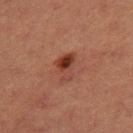  biopsy_status: not biopsied; imaged during a skin examination
  lesion_size:
    long_diameter_mm_approx: 3.5
  lighting: cross-polarized
  patient:
    sex: female
    age_approx: 40
  site: right thigh
  image:
    source: total-body photography crop
    field_of_view_mm: 15
  automated_metrics:
    eccentricity: 0.75
    shape_asymmetry: 0.4
    vs_skin_darker_L: 8.0
    nevus_likeness_0_100: 85
    lesion_detection_confidence_0_100: 100